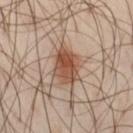  biopsy_status: not biopsied; imaged during a skin examination
  image:
    source: total-body photography crop
    field_of_view_mm: 15
  patient:
    sex: male
    age_approx: 40
  site: right thigh